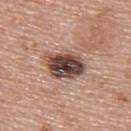The recorded lesion diameter is about 5 mm. A 15 mm crop from a total-body photograph taken for skin-cancer surveillance. A female subject, about 60 years old. Located on the upper back.